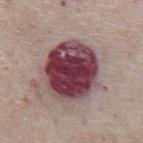notes: no biopsy performed (imaged during a skin exam)
acquisition: total-body-photography crop, ~15 mm field of view
lighting: white-light illumination
anatomic site: the front of the torso
diameter: ≈7 mm
patient: male, aged 73 to 77